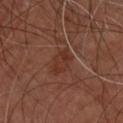follow-up=no biopsy performed (imaged during a skin exam) | acquisition=~15 mm tile from a whole-body skin photo | anatomic site=the upper back | patient=male, in their mid- to late 40s | lesion size=about 3 mm | illumination=cross-polarized illumination.